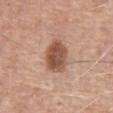biopsy status: no biopsy performed (imaged during a skin exam) | subject: male, aged 63 to 67 | size: about 4 mm | imaging modality: 15 mm crop, total-body photography | body site: the front of the torso.